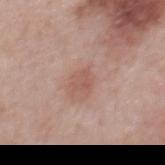notes: no biopsy performed (imaged during a skin exam) | tile lighting: white-light illumination | subject: male, aged 63–67 | body site: the mid back | image source: 15 mm crop, total-body photography | automated lesion analysis: an area of roughly 4 mm² and an eccentricity of roughly 0.6.